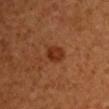Image and clinical context:
The lesion-visualizer software estimated an automated nevus-likeness rating near 95 out of 100 and lesion-presence confidence of about 100/100. A male subject, aged approximately 45. Captured under cross-polarized illumination. A close-up tile cropped from a whole-body skin photograph, about 15 mm across. From the left upper arm.